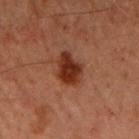No biopsy was performed on this lesion — it was imaged during a full skin examination and was not determined to be concerning. The lesion is on the front of the torso. A close-up tile cropped from a whole-body skin photograph, about 15 mm across. A male patient, in their 60s. Measured at roughly 3.5 mm in maximum diameter. The tile uses cross-polarized illumination.